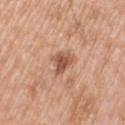Captured during whole-body skin photography for melanoma surveillance; the lesion was not biopsied.
A roughly 15 mm field-of-view crop from a total-body skin photograph.
From the left upper arm.
This is a white-light tile.
The lesion's longest dimension is about 3 mm.
An algorithmic analysis of the crop reported a lesion area of about 5.5 mm² and an outline eccentricity of about 0.6 (0 = round, 1 = elongated). And it measured a border-irregularity rating of about 4.5/10, a within-lesion color-variation index near 3.5/10, and radial color variation of about 1.
A male patient, in their 50s.